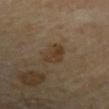Q: Was this lesion biopsied?
A: no biopsy performed (imaged during a skin exam)
Q: Automated lesion metrics?
A: a lesion area of about 6 mm² and two-axis asymmetry of about 0.25; a lesion color around L≈35 a*≈14 b*≈28 in CIELAB, a lesion–skin lightness drop of about 7, and a lesion-to-skin contrast of about 7.5 (normalized; higher = more distinct)
Q: What kind of image is this?
A: total-body-photography crop, ~15 mm field of view
Q: Lesion location?
A: the arm
Q: Illumination type?
A: cross-polarized
Q: Who is the patient?
A: male, aged around 70